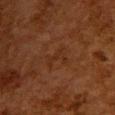Clinical impression:
This lesion was catalogued during total-body skin photography and was not selected for biopsy.
Acquisition and patient details:
This is a cross-polarized tile. The subject is a female roughly 50 years of age. The recorded lesion diameter is about 3 mm. A region of skin cropped from a whole-body photographic capture, roughly 15 mm wide. Located on the upper back.